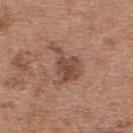Impression: The lesion was tiled from a total-body skin photograph and was not biopsied. Context: The lesion is on the upper back. Imaged with white-light lighting. The lesion's longest dimension is about 5 mm. A female patient, in their 40s. Automated image analysis of the tile measured a mean CIELAB color near L≈47 a*≈21 b*≈27 and about 10 CIELAB-L* units darker than the surrounding skin. It also reported a border-irregularity index near 6.5/10, internal color variation of about 3.5 on a 0–10 scale, and radial color variation of about 1.5. A 15 mm close-up extracted from a 3D total-body photography capture.